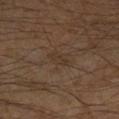notes = no biopsy performed (imaged during a skin exam)
body site = the leg
lesion size = about 3.5 mm
imaging modality = ~15 mm crop, total-body skin-cancer survey
subject = male, aged 58 to 62